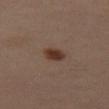Located on the left thigh.
A region of skin cropped from a whole-body photographic capture, roughly 15 mm wide.
A female subject aged 38 to 42.